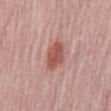Case summary:
– biopsy status · catalogued during a skin exam; not biopsied
– site · the back
– image-analysis metrics · an area of roughly 8 mm², an outline eccentricity of about 0.65 (0 = round, 1 = elongated), and a symmetry-axis asymmetry near 0.2; border irregularity of about 2 on a 0–10 scale and a peripheral color-asymmetry measure near 1; an automated nevus-likeness rating near 100 out of 100 and lesion-presence confidence of about 100/100
– patient · female, aged around 65
– lighting · white-light illumination
– lesion diameter · ~3.5 mm (longest diameter)
– acquisition · ~15 mm tile from a whole-body skin photo